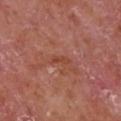Part of a total-body skin-imaging series; this lesion was reviewed on a skin check and was not flagged for biopsy.
Longest diameter approximately 2.5 mm.
Automated tile analysis of the lesion measured an area of roughly 2 mm², an eccentricity of roughly 0.95, and a symmetry-axis asymmetry near 0.4. And it measured a mean CIELAB color near L≈45 a*≈28 b*≈32, roughly 6 lightness units darker than nearby skin, and a normalized lesion–skin contrast near 6. The software also gave a border-irregularity rating of about 4/10, a color-variation rating of about 0/10, and peripheral color asymmetry of about 0.
On the chest.
A male subject in their mid- to late 60s.
A 15 mm close-up extracted from a 3D total-body photography capture.